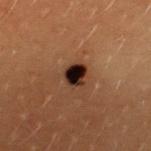<tbp_lesion>
  <biopsy_status>not biopsied; imaged during a skin examination</biopsy_status>
  <lighting>cross-polarized</lighting>
  <patient>
    <sex>female</sex>
    <age_approx>20</age_approx>
  </patient>
  <site>upper back</site>
  <lesion_size>
    <long_diameter_mm_approx>2.5</long_diameter_mm_approx>
  </lesion_size>
  <image>
    <source>total-body photography crop</source>
    <field_of_view_mm>15</field_of_view_mm>
  </image>
</tbp_lesion>